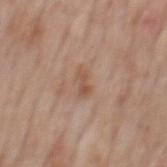Assessment: This lesion was catalogued during total-body skin photography and was not selected for biopsy. Background: A male patient in their mid-70s. A 15 mm close-up tile from a total-body photography series done for melanoma screening. The tile uses white-light illumination. Approximately 3 mm at its widest. The lesion is located on the back.